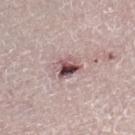Clinical summary:
This image is a 15 mm lesion crop taken from a total-body photograph. The patient is a male aged 63–67. The tile uses white-light illumination. The lesion is on the right lower leg.